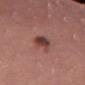Notes:
* notes — no biopsy performed (imaged during a skin exam)
* automated metrics — a lesion area of about 5 mm², an outline eccentricity of about 0.85 (0 = round, 1 = elongated), and a symmetry-axis asymmetry near 0.2; an average lesion color of about L≈40 a*≈23 b*≈23 (CIELAB), a lesion–skin lightness drop of about 13, and a normalized lesion–skin contrast near 10; a border-irregularity index near 2/10, a within-lesion color-variation index near 5.5/10, and a peripheral color-asymmetry measure near 1.5; a classifier nevus-likeness of about 90/100 and lesion-presence confidence of about 100/100
* lighting — white-light
* lesion diameter — ~3.5 mm (longest diameter)
* image source — ~15 mm tile from a whole-body skin photo
* location — the left thigh
* patient — female, approximately 50 years of age